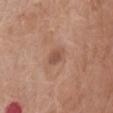Impression:
Captured during whole-body skin photography for melanoma surveillance; the lesion was not biopsied.
Context:
The subject is a female aged around 75. This is a white-light tile. On the abdomen. A lesion tile, about 15 mm wide, cut from a 3D total-body photograph. About 2.5 mm across. The total-body-photography lesion software estimated a mean CIELAB color near L≈52 a*≈22 b*≈28 and roughly 9 lightness units darker than nearby skin.